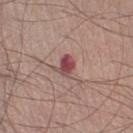Assessment:
Part of a total-body skin-imaging series; this lesion was reviewed on a skin check and was not flagged for biopsy.
Acquisition and patient details:
About 2.5 mm across. A region of skin cropped from a whole-body photographic capture, roughly 15 mm wide. Imaged with white-light lighting. The subject is a male aged 53 to 57.